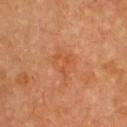  biopsy_status: not biopsied; imaged during a skin examination
  lesion_size:
    long_diameter_mm_approx: 3.0
  site: front of the torso
  lighting: cross-polarized
  automated_metrics:
    cielab_L: 55
    cielab_a: 30
    cielab_b: 41
    vs_skin_darker_L: 6.0
    vs_skin_contrast_norm: 5.0
    nevus_likeness_0_100: 0
    lesion_detection_confidence_0_100: 100
  image:
    source: total-body photography crop
    field_of_view_mm: 15